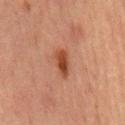Assessment: Imaged during a routine full-body skin examination; the lesion was not biopsied and no histopathology is available. Context: A male patient, aged around 65. Automated image analysis of the tile measured a lesion area of about 5 mm², an outline eccentricity of about 0.85 (0 = round, 1 = elongated), and two-axis asymmetry of about 0.25. And it measured border irregularity of about 3 on a 0–10 scale, internal color variation of about 3 on a 0–10 scale, and radial color variation of about 1. The lesion is on the abdomen. This image is a 15 mm lesion crop taken from a total-body photograph.